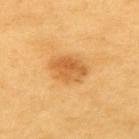<record>
<biopsy_status>not biopsied; imaged during a skin examination</biopsy_status>
<lighting>cross-polarized</lighting>
<image>
  <source>total-body photography crop</source>
  <field_of_view_mm>15</field_of_view_mm>
</image>
<patient>
  <sex>male</sex>
  <age_approx>60</age_approx>
</patient>
<site>upper back</site>
</record>